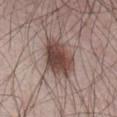| feature | finding |
|---|---|
| follow-up | total-body-photography surveillance lesion; no biopsy |
| illumination | white-light |
| automated lesion analysis | an area of roughly 14 mm²; a mean CIELAB color near L≈44 a*≈18 b*≈21, roughly 15 lightness units darker than nearby skin, and a normalized border contrast of about 11 |
| lesion size | about 5.5 mm |
| body site | the front of the torso |
| image | ~15 mm tile from a whole-body skin photo |
| patient | male, aged 63 to 67 |